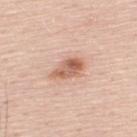notes = total-body-photography surveillance lesion; no biopsy | patient = male, aged 48–52 | automated lesion analysis = a footprint of about 7 mm², a shape eccentricity near 0.85, and two-axis asymmetry of about 0.3; a mean CIELAB color near L≈62 a*≈23 b*≈31, about 13 CIELAB-L* units darker than the surrounding skin, and a normalized lesion–skin contrast near 8.5; a border-irregularity rating of about 3/10 and radial color variation of about 2 | image source = 15 mm crop, total-body photography | anatomic site = the upper back | diameter = about 4 mm.